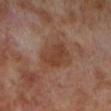Clinical impression: Captured during whole-body skin photography for melanoma surveillance; the lesion was not biopsied. Context: A male subject, in their 70s. A close-up tile cropped from a whole-body skin photograph, about 15 mm across. The lesion-visualizer software estimated an area of roughly 13 mm², a shape eccentricity near 0.65, and a symmetry-axis asymmetry near 0.2. The analysis additionally found an average lesion color of about L≈40 a*≈20 b*≈28 (CIELAB), roughly 8 lightness units darker than nearby skin, and a lesion-to-skin contrast of about 7 (normalized; higher = more distinct). The analysis additionally found a classifier nevus-likeness of about 10/100 and a detector confidence of about 100 out of 100 that the crop contains a lesion. The lesion is on the left lower leg. Longest diameter approximately 4.5 mm. Imaged with cross-polarized lighting.